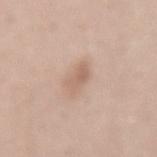Imaged during a routine full-body skin examination; the lesion was not biopsied and no histopathology is available.
Longest diameter approximately 3.5 mm.
A roughly 15 mm field-of-view crop from a total-body skin photograph.
The subject is a female in their mid-30s.
The lesion is on the abdomen.
Automated tile analysis of the lesion measured a lesion area of about 5 mm², an eccentricity of roughly 0.85, and a symmetry-axis asymmetry near 0.3. It also reported a lesion color around L≈62 a*≈17 b*≈28 in CIELAB, roughly 8 lightness units darker than nearby skin, and a normalized border contrast of about 5.5. The analysis additionally found a nevus-likeness score of about 15/100.
Imaged with white-light lighting.